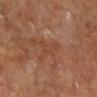Impression: No biopsy was performed on this lesion — it was imaged during a full skin examination and was not determined to be concerning. Context: This is a cross-polarized tile. On the right lower leg. The subject is a female aged around 40. Longest diameter approximately 3.5 mm. A 15 mm crop from a total-body photograph taken for skin-cancer surveillance.